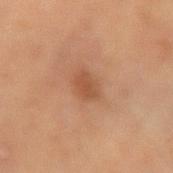Part of a total-body skin-imaging series; this lesion was reviewed on a skin check and was not flagged for biopsy. A 15 mm close-up tile from a total-body photography series done for melanoma screening. Longest diameter approximately 2.5 mm. The subject is a female aged 78–82. The lesion is on the left upper arm.